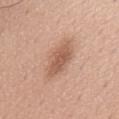Measured at roughly 5.5 mm in maximum diameter. A male patient, about 55 years old. Cropped from a total-body skin-imaging series; the visible field is about 15 mm. This is a white-light tile.